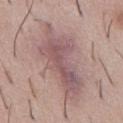biopsy status — catalogued during a skin exam; not biopsied
patient — male, aged 48 to 52
body site — the abdomen
TBP lesion metrics — a classifier nevus-likeness of about 0/100 and lesion-presence confidence of about 55/100
tile lighting — white-light
image source — 15 mm crop, total-body photography
lesion diameter — about 10 mm